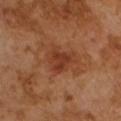Q: Was this lesion biopsied?
A: catalogued during a skin exam; not biopsied
Q: What is the imaging modality?
A: ~15 mm tile from a whole-body skin photo
Q: Who is the patient?
A: male, about 65 years old